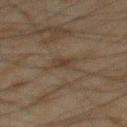Clinical impression: This lesion was catalogued during total-body skin photography and was not selected for biopsy. Background: Measured at roughly 2.5 mm in maximum diameter. From the back. A region of skin cropped from a whole-body photographic capture, roughly 15 mm wide. The tile uses cross-polarized illumination. The subject is a male about 45 years old. Automated image analysis of the tile measured an area of roughly 3 mm², an outline eccentricity of about 0.85 (0 = round, 1 = elongated), and two-axis asymmetry of about 0.25.